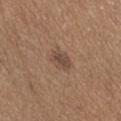notes: no biopsy performed (imaged during a skin exam)
imaging modality: ~15 mm crop, total-body skin-cancer survey
patient: male, about 65 years old
location: the abdomen